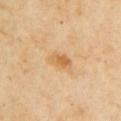The lesion was tiled from a total-body skin photograph and was not biopsied.
The lesion is on the left upper arm.
The patient is a female aged approximately 60.
The recorded lesion diameter is about 3 mm.
Automated tile analysis of the lesion measured a shape eccentricity near 0.8 and a symmetry-axis asymmetry near 0.2. It also reported a lesion color around L≈63 a*≈20 b*≈43 in CIELAB.
A region of skin cropped from a whole-body photographic capture, roughly 15 mm wide.